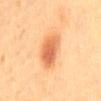Q: Was a biopsy performed?
A: imaged on a skin check; not biopsied
Q: Who is the patient?
A: female, aged 43–47
Q: Lesion size?
A: ≈4.5 mm
Q: What kind of image is this?
A: ~15 mm tile from a whole-body skin photo
Q: What is the anatomic site?
A: the mid back